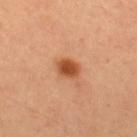Clinical impression:
Recorded during total-body skin imaging; not selected for excision or biopsy.
Image and clinical context:
A male patient, approximately 55 years of age. Longest diameter approximately 2.5 mm. A 15 mm crop from a total-body photograph taken for skin-cancer surveillance. The tile uses cross-polarized illumination. On the mid back.